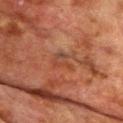Part of a total-body skin-imaging series; this lesion was reviewed on a skin check and was not flagged for biopsy. The tile uses cross-polarized illumination. A male patient aged 73–77. Located on the front of the torso. Automated tile analysis of the lesion measured a lesion color around L≈37 a*≈22 b*≈28 in CIELAB and roughly 5 lightness units darker than nearby skin. The software also gave a border-irregularity index near 5/10 and radial color variation of about 1.5. Cropped from a total-body skin-imaging series; the visible field is about 15 mm. The recorded lesion diameter is about 3 mm.